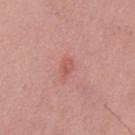A roughly 15 mm field-of-view crop from a total-body skin photograph.
The lesion is on the mid back.
A male subject roughly 50 years of age.
Captured under white-light illumination.